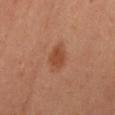This lesion was catalogued during total-body skin photography and was not selected for biopsy. An algorithmic analysis of the crop reported roughly 7 lightness units darker than nearby skin and a normalized lesion–skin contrast near 6.5. It also reported lesion-presence confidence of about 100/100. This image is a 15 mm lesion crop taken from a total-body photograph. Longest diameter approximately 3.5 mm. Located on the front of the torso. A male subject approximately 40 years of age.